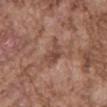No biopsy was performed on this lesion — it was imaged during a full skin examination and was not determined to be concerning.
Cropped from a whole-body photographic skin survey; the tile spans about 15 mm.
A male subject about 75 years old.
The recorded lesion diameter is about 3.5 mm.
This is a white-light tile.
The lesion is on the back.